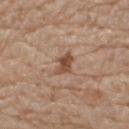Findings:
* tile lighting · white-light illumination
* subject · male, about 80 years old
* lesion diameter · ≈3 mm
* body site · the upper back
* imaging modality · ~15 mm tile from a whole-body skin photo